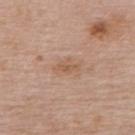{
  "biopsy_status": "not biopsied; imaged during a skin examination",
  "lesion_size": {
    "long_diameter_mm_approx": 2.5
  },
  "automated_metrics": {
    "area_mm2_approx": 3.0,
    "eccentricity": 0.85,
    "shape_asymmetry": 0.25,
    "cielab_L": 56,
    "cielab_a": 19,
    "cielab_b": 31,
    "vs_skin_darker_L": 7.0,
    "vs_skin_contrast_norm": 5.5,
    "border_irregularity_0_10": 2.5,
    "color_variation_0_10": 1.0,
    "peripheral_color_asymmetry": 0.0
  },
  "lighting": "white-light",
  "patient": {
    "sex": "female",
    "age_approx": 50
  },
  "site": "upper back",
  "image": {
    "source": "total-body photography crop",
    "field_of_view_mm": 15
  }
}